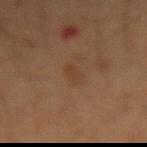Recorded during total-body skin imaging; not selected for excision or biopsy. A region of skin cropped from a whole-body photographic capture, roughly 15 mm wide. Located on the abdomen. This is a cross-polarized tile. Automated image analysis of the tile measured a footprint of about 3 mm², an eccentricity of roughly 0.9, and a shape-asymmetry score of about 0.3 (0 = symmetric). And it measured a nevus-likeness score of about 5/100 and lesion-presence confidence of about 100/100. A male patient, approximately 55 years of age.